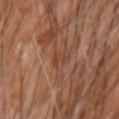Impression:
No biopsy was performed on this lesion — it was imaged during a full skin examination and was not determined to be concerning.
Image and clinical context:
The lesion is on the right forearm. About 3 mm across. A roughly 15 mm field-of-view crop from a total-body skin photograph. Automated image analysis of the tile measured an area of roughly 2.5 mm² and two-axis asymmetry of about 0.55. And it measured an automated nevus-likeness rating near 0 out of 100 and lesion-presence confidence of about 65/100. A male subject, approximately 65 years of age. Imaged with cross-polarized lighting.